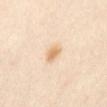| key | value |
|---|---|
| workup | no biopsy performed (imaged during a skin exam) |
| lighting | cross-polarized illumination |
| imaging modality | ~15 mm tile from a whole-body skin photo |
| patient | female, about 40 years old |
| body site | the abdomen |
| TBP lesion metrics | a classifier nevus-likeness of about 95/100 and a lesion-detection confidence of about 100/100 |
| lesion diameter | ≈2.5 mm |